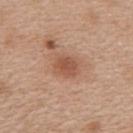– biopsy status · catalogued during a skin exam; not biopsied
– TBP lesion metrics · a footprint of about 5.5 mm², a shape eccentricity near 0.6, and a symmetry-axis asymmetry near 0.15; a border-irregularity index near 1.5/10 and a peripheral color-asymmetry measure near 1
– imaging modality · ~15 mm crop, total-body skin-cancer survey
– body site · the upper back
– subject · female, aged around 40